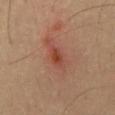Assessment: Recorded during total-body skin imaging; not selected for excision or biopsy. Context: Measured at roughly 3.5 mm in maximum diameter. A male subject, about 55 years old. A close-up tile cropped from a whole-body skin photograph, about 15 mm across. Automated image analysis of the tile measured a lesion area of about 4.5 mm², an outline eccentricity of about 0.85 (0 = round, 1 = elongated), and a shape-asymmetry score of about 0.25 (0 = symmetric). Located on the mid back. Imaged with cross-polarized lighting.